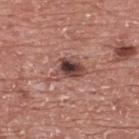No biopsy was performed on this lesion — it was imaged during a full skin examination and was not determined to be concerning.
A male patient, aged 68–72.
The lesion is located on the upper back.
The recorded lesion diameter is about 4 mm.
Cropped from a total-body skin-imaging series; the visible field is about 15 mm.
An algorithmic analysis of the crop reported an outline eccentricity of about 0.75 (0 = round, 1 = elongated).